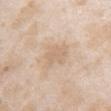workup: imaged on a skin check; not biopsied
imaging modality: 15 mm crop, total-body photography
subject: female, aged 23–27
lighting: white-light
body site: the chest
automated metrics: an outline eccentricity of about 0.45 (0 = round, 1 = elongated) and two-axis asymmetry of about 0.3; a border-irregularity rating of about 3.5/10, internal color variation of about 1.5 on a 0–10 scale, and peripheral color asymmetry of about 0.5; a classifier nevus-likeness of about 0/100 and a detector confidence of about 100 out of 100 that the crop contains a lesion
lesion size: about 3.5 mm